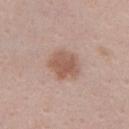No biopsy was performed on this lesion — it was imaged during a full skin examination and was not determined to be concerning. A 15 mm close-up tile from a total-body photography series done for melanoma screening. From the chest. A female patient, aged around 35.